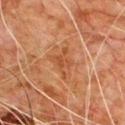Imaged during a routine full-body skin examination; the lesion was not biopsied and no histopathology is available.
Automated image analysis of the tile measured lesion-presence confidence of about 100/100.
The lesion is located on the chest.
A male subject, aged 78–82.
A roughly 15 mm field-of-view crop from a total-body skin photograph.
The tile uses cross-polarized illumination.
About 4 mm across.